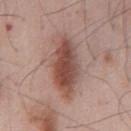workup = total-body-photography surveillance lesion; no biopsy | subject = male, aged around 55 | lesion size = ≈7.5 mm | image = ~15 mm crop, total-body skin-cancer survey | location = the chest.